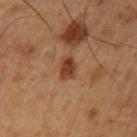Impression: Recorded during total-body skin imaging; not selected for excision or biopsy. Context: Located on the left upper arm. Imaged with cross-polarized lighting. Automated tile analysis of the lesion measured an area of roughly 4.5 mm². A close-up tile cropped from a whole-body skin photograph, about 15 mm across. A male patient aged 48–52. About 3.5 mm across.